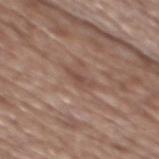Clinical impression: Recorded during total-body skin imaging; not selected for excision or biopsy. Background: The recorded lesion diameter is about 3 mm. Automated tile analysis of the lesion measured a lesion–skin lightness drop of about 7 and a lesion-to-skin contrast of about 5.5 (normalized; higher = more distinct). The software also gave border irregularity of about 5.5 on a 0–10 scale, a color-variation rating of about 0/10, and radial color variation of about 0. And it measured an automated nevus-likeness rating near 0 out of 100. On the mid back. Cropped from a whole-body photographic skin survey; the tile spans about 15 mm. A male patient roughly 75 years of age.